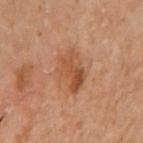Automated tile analysis of the lesion measured a footprint of about 10 mm² and two-axis asymmetry of about 0.25. And it measured a nevus-likeness score of about 50/100 and a lesion-detection confidence of about 100/100. The patient is a female in their 60s. Imaged with cross-polarized lighting. Cropped from a total-body skin-imaging series; the visible field is about 15 mm. The lesion is located on the left arm.